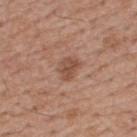Clinical impression:
Captured during whole-body skin photography for melanoma surveillance; the lesion was not biopsied.
Acquisition and patient details:
Located on the upper back. This image is a 15 mm lesion crop taken from a total-body photograph. Automated tile analysis of the lesion measured a lesion area of about 4.5 mm², an outline eccentricity of about 0.7 (0 = round, 1 = elongated), and two-axis asymmetry of about 0.3. The software also gave a within-lesion color-variation index near 2.5/10 and radial color variation of about 1. This is a white-light tile. The subject is a male approximately 50 years of age.